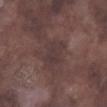No biopsy was performed on this lesion — it was imaged during a full skin examination and was not determined to be concerning.
Automated tile analysis of the lesion measured a lesion area of about 9.5 mm², an outline eccentricity of about 0.75 (0 = round, 1 = elongated), and two-axis asymmetry of about 0.25. It also reported a border-irregularity rating of about 3/10, a color-variation rating of about 2/10, and peripheral color asymmetry of about 0.5. And it measured a classifier nevus-likeness of about 0/100.
Longest diameter approximately 4 mm.
The tile uses white-light illumination.
The lesion is located on the right lower leg.
The subject is a male aged 73–77.
A close-up tile cropped from a whole-body skin photograph, about 15 mm across.